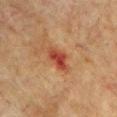Q: Is there a histopathology result?
A: catalogued during a skin exam; not biopsied
Q: Who is the patient?
A: male, roughly 75 years of age
Q: How was this image acquired?
A: ~15 mm crop, total-body skin-cancer survey
Q: Lesion location?
A: the left upper arm